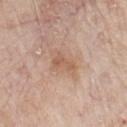Imaged during a routine full-body skin examination; the lesion was not biopsied and no histopathology is available. The lesion is on the chest. This is a white-light tile. Approximately 3 mm at its widest. A close-up tile cropped from a whole-body skin photograph, about 15 mm across. A male patient about 80 years old. Automated image analysis of the tile measured radial color variation of about 0.5.